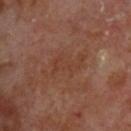{"biopsy_status": "not biopsied; imaged during a skin examination", "lighting": "cross-polarized", "automated_metrics": {"area_mm2_approx": 9.0, "eccentricity": 0.9, "shape_asymmetry": 0.5, "cielab_L": 38, "cielab_a": 21, "cielab_b": 28, "vs_skin_darker_L": 5.0, "vs_skin_contrast_norm": 4.5, "color_variation_0_10": 1.5, "peripheral_color_asymmetry": 0.5, "nevus_likeness_0_100": 0, "lesion_detection_confidence_0_100": 100}, "image": {"source": "total-body photography crop", "field_of_view_mm": 15}, "site": "upper back", "patient": {"sex": "male", "age_approx": 70}, "lesion_size": {"long_diameter_mm_approx": 5.5}}